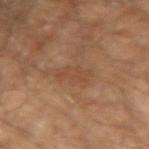From the right upper arm.
Captured under cross-polarized illumination.
A male patient, aged 63 to 67.
This image is a 15 mm lesion crop taken from a total-body photograph.